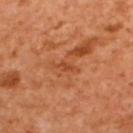Impression:
This lesion was catalogued during total-body skin photography and was not selected for biopsy.
Background:
Automated image analysis of the tile measured a lesion–skin lightness drop of about 8 and a normalized lesion–skin contrast near 5.5. The analysis additionally found border irregularity of about 4 on a 0–10 scale, a color-variation rating of about 0/10, and radial color variation of about 0. And it measured a nevus-likeness score of about 0/100 and a lesion-detection confidence of about 100/100. About 1.5 mm across. The subject is a female aged 53–57. This image is a 15 mm lesion crop taken from a total-body photograph. The tile uses cross-polarized illumination. Located on the upper back.